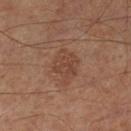workup = imaged on a skin check; not biopsied | patient = male, about 55 years old | body site = the left lower leg | image = ~15 mm tile from a whole-body skin photo | lesion diameter = ≈4.5 mm | tile lighting = cross-polarized | TBP lesion metrics = a lesion area of about 9 mm², a shape eccentricity near 0.7, and a symmetry-axis asymmetry near 0.25; a mean CIELAB color near L≈42 a*≈20 b*≈27, roughly 7 lightness units darker than nearby skin, and a normalized border contrast of about 5.5; border irregularity of about 3 on a 0–10 scale, a within-lesion color-variation index near 2/10, and a peripheral color-asymmetry measure near 0.5; a nevus-likeness score of about 10/100 and lesion-presence confidence of about 100/100.